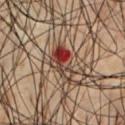Impression:
The lesion was photographed on a routine skin check and not biopsied; there is no pathology result.